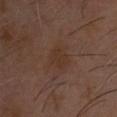size: about 3 mm | subject: male, aged 68–72 | site: the head or neck | imaging modality: ~15 mm crop, total-body skin-cancer survey | image-analysis metrics: an area of roughly 5.5 mm² and two-axis asymmetry of about 0.25; a lesion color around L≈30 a*≈16 b*≈24 in CIELAB and about 4 CIELAB-L* units darker than the surrounding skin.